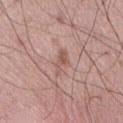Q: Where on the body is the lesion?
A: the abdomen
Q: What is the imaging modality?
A: ~15 mm crop, total-body skin-cancer survey
Q: Lesion size?
A: ~3.5 mm (longest diameter)
Q: Illumination type?
A: white-light illumination
Q: What are the patient's age and sex?
A: male, aged 53 to 57